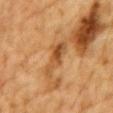follow-up = imaged on a skin check; not biopsied
acquisition = ~15 mm tile from a whole-body skin photo
patient = male, about 85 years old or older
location = the chest